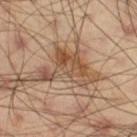Imaged during a routine full-body skin examination; the lesion was not biopsied and no histopathology is available.
Located on the leg.
A male patient aged around 40.
A lesion tile, about 15 mm wide, cut from a 3D total-body photograph.
This is a cross-polarized tile.